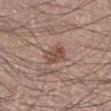notes — catalogued during a skin exam; not biopsied | size — ≈2.5 mm | site — the left lower leg | automated lesion analysis — a mean CIELAB color near L≈49 a*≈20 b*≈25 and roughly 10 lightness units darker than nearby skin; a border-irregularity rating of about 2/10, a within-lesion color-variation index near 3/10, and peripheral color asymmetry of about 1; a detector confidence of about 100 out of 100 that the crop contains a lesion | image — ~15 mm crop, total-body skin-cancer survey | subject — male, aged approximately 30.